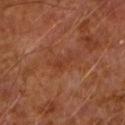{
  "site": "arm",
  "lighting": "cross-polarized",
  "image": {
    "source": "total-body photography crop",
    "field_of_view_mm": 15
  },
  "patient": {
    "sex": "male",
    "age_approx": 65
  },
  "lesion_size": {
    "long_diameter_mm_approx": 2.5
  }
}